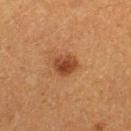Recorded during total-body skin imaging; not selected for excision or biopsy. The lesion is on the left thigh. A 15 mm crop from a total-body photograph taken for skin-cancer surveillance. Imaged with cross-polarized lighting. A female subject aged 38–42.